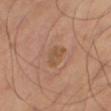site: the leg
diameter: ≈3 mm
acquisition: total-body-photography crop, ~15 mm field of view
lighting: cross-polarized illumination
TBP lesion metrics: an area of roughly 5 mm², an eccentricity of roughly 0.75, and a shape-asymmetry score of about 0.2 (0 = symmetric); border irregularity of about 2 on a 0–10 scale, a color-variation rating of about 3/10, and radial color variation of about 1; a nevus-likeness score of about 0/100